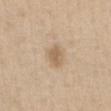Notes:
• notes · no biopsy performed (imaged during a skin exam)
• image · ~15 mm crop, total-body skin-cancer survey
• location · the chest
• lesion diameter · about 2.5 mm
• patient · female, approximately 60 years of age
• illumination · white-light illumination